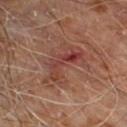biopsy_status: not biopsied; imaged during a skin examination
automated_metrics:
  nevus_likeness_0_100: 0
  lesion_detection_confidence_0_100: 100
lesion_size:
  long_diameter_mm_approx: 5.0
patient:
  sex: male
  age_approx: 70
site: left lower leg
image:
  source: total-body photography crop
  field_of_view_mm: 15